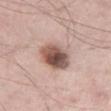The lesion was photographed on a routine skin check and not biopsied; there is no pathology result. A close-up tile cropped from a whole-body skin photograph, about 15 mm across. Automated image analysis of the tile measured an area of roughly 16 mm², an outline eccentricity of about 0.75 (0 = round, 1 = elongated), and a symmetry-axis asymmetry near 0.15. And it measured a lesion color around L≈57 a*≈18 b*≈24 in CIELAB and a normalized lesion–skin contrast near 9.5. And it measured border irregularity of about 2 on a 0–10 scale, a color-variation rating of about 10/10, and peripheral color asymmetry of about 4. On the right thigh. The patient is a male aged around 55.